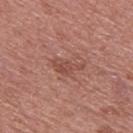Captured during whole-body skin photography for melanoma surveillance; the lesion was not biopsied. A 15 mm close-up extracted from a 3D total-body photography capture. On the upper back. Captured under white-light illumination. A male patient approximately 55 years of age.